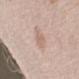notes = imaged on a skin check; not biopsied | site = the left forearm | image source = ~15 mm tile from a whole-body skin photo | automated metrics = a lesion area of about 3 mm² and a symmetry-axis asymmetry near 0.3; a border-irregularity rating of about 3/10, a within-lesion color-variation index near 1/10, and peripheral color asymmetry of about 0 | size = ≈2.5 mm | subject = female, roughly 50 years of age | illumination = white-light.